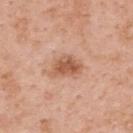follow-up: catalogued during a skin exam; not biopsied
patient: female, aged 48–52
automated lesion analysis: a lesion area of about 8 mm², a shape eccentricity near 0.75, and a shape-asymmetry score of about 0.3 (0 = symmetric); a border-irregularity index near 2.5/10, a color-variation rating of about 4/10, and a peripheral color-asymmetry measure near 1.5
site: the back
diameter: ~4 mm (longest diameter)
illumination: white-light
image: ~15 mm crop, total-body skin-cancer survey